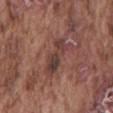Q: Was this lesion biopsied?
A: total-body-photography surveillance lesion; no biopsy
Q: Lesion size?
A: about 5 mm
Q: Lesion location?
A: the mid back
Q: Who is the patient?
A: male, in their mid-70s
Q: Illumination type?
A: white-light illumination
Q: What is the imaging modality?
A: ~15 mm crop, total-body skin-cancer survey